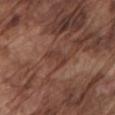Assessment: The lesion was tiled from a total-body skin photograph and was not biopsied. Clinical summary: The tile uses white-light illumination. The recorded lesion diameter is about 3 mm. A male patient, approximately 75 years of age. The total-body-photography lesion software estimated a classifier nevus-likeness of about 0/100 and lesion-presence confidence of about 80/100. Cropped from a total-body skin-imaging series; the visible field is about 15 mm. The lesion is located on the left upper arm.